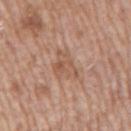biopsy status: no biopsy performed (imaged during a skin exam) | anatomic site: the right upper arm | image source: ~15 mm tile from a whole-body skin photo | subject: male, aged approximately 70.